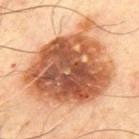Assessment:
Part of a total-body skin-imaging series; this lesion was reviewed on a skin check and was not flagged for biopsy.
Clinical summary:
A male patient, in their 70s. Measured at roughly 10 mm in maximum diameter. This is a cross-polarized tile. A roughly 15 mm field-of-view crop from a total-body skin photograph. The lesion is on the chest. The total-body-photography lesion software estimated roughly 17 lightness units darker than nearby skin. The analysis additionally found border irregularity of about 3 on a 0–10 scale, a color-variation rating of about 8.5/10, and a peripheral color-asymmetry measure near 2.5. The software also gave an automated nevus-likeness rating near 85 out of 100 and a detector confidence of about 100 out of 100 that the crop contains a lesion.